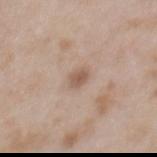No biopsy was performed on this lesion — it was imaged during a full skin examination and was not determined to be concerning. The lesion is located on the mid back. Imaged with white-light lighting. The total-body-photography lesion software estimated a lesion area of about 3 mm² and two-axis asymmetry of about 0.3. It also reported border irregularity of about 2.5 on a 0–10 scale, internal color variation of about 1.5 on a 0–10 scale, and a peripheral color-asymmetry measure near 0.5. The software also gave a classifier nevus-likeness of about 20/100 and a detector confidence of about 100 out of 100 that the crop contains a lesion. A female patient, approximately 30 years of age. A 15 mm close-up tile from a total-body photography series done for melanoma screening.